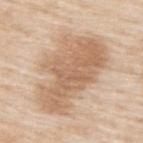biopsy_status: not biopsied; imaged during a skin examination
lighting: white-light
patient:
  sex: male
  age_approx: 80
site: upper back
lesion_size:
  long_diameter_mm_approx: 10.0
image:
  source: total-body photography crop
  field_of_view_mm: 15